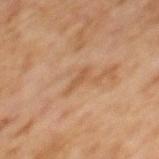| feature | finding |
|---|---|
| biopsy status | imaged on a skin check; not biopsied |
| acquisition | total-body-photography crop, ~15 mm field of view |
| size | about 2.5 mm |
| illumination | cross-polarized illumination |
| subject | female, in their 60s |
| anatomic site | the upper back |